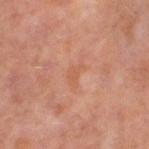| key | value |
|---|---|
| notes | catalogued during a skin exam; not biopsied |